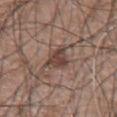No biopsy was performed on this lesion — it was imaged during a full skin examination and was not determined to be concerning. Approximately 4 mm at its widest. The subject is a male aged 58 to 62. A 15 mm close-up extracted from a 3D total-body photography capture. This is a white-light tile. An algorithmic analysis of the crop reported a footprint of about 5.5 mm² and a shape eccentricity near 0.8. The software also gave a mean CIELAB color near L≈42 a*≈17 b*≈23, about 11 CIELAB-L* units darker than the surrounding skin, and a lesion-to-skin contrast of about 9 (normalized; higher = more distinct). The analysis additionally found a classifier nevus-likeness of about 10/100. The lesion is on the chest.